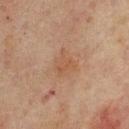Impression: Part of a total-body skin-imaging series; this lesion was reviewed on a skin check and was not flagged for biopsy. Acquisition and patient details: Measured at roughly 3.5 mm in maximum diameter. A roughly 15 mm field-of-view crop from a total-body skin photograph. The subject is a male approximately 65 years of age. Imaged with cross-polarized lighting. Located on the mid back.